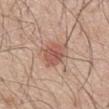Notes:
– workup — catalogued during a skin exam; not biopsied
– lesion size — about 3.5 mm
– lighting — white-light
– automated metrics — a footprint of about 8.5 mm², an outline eccentricity of about 0.6 (0 = round, 1 = elongated), and two-axis asymmetry of about 0.2; a normalized border contrast of about 7; a color-variation rating of about 3/10 and a peripheral color-asymmetry measure near 1
– patient — male, about 50 years old
– site — the mid back
– image — 15 mm crop, total-body photography